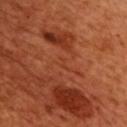This is a cross-polarized tile. The lesion is on the chest. A male patient, about 50 years old. A 15 mm crop from a total-body photograph taken for skin-cancer surveillance. About 1.5 mm across.